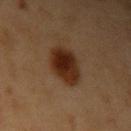{
  "biopsy_status": "not biopsied; imaged during a skin examination",
  "image": {
    "source": "total-body photography crop",
    "field_of_view_mm": 15
  },
  "site": "arm",
  "patient": {
    "sex": "female",
    "age_approx": 40
  },
  "lesion_size": {
    "long_diameter_mm_approx": 4.0
  },
  "automated_metrics": {
    "area_mm2_approx": 12.0,
    "eccentricity": 0.5,
    "shape_asymmetry": 0.1,
    "cielab_L": 23,
    "cielab_a": 17,
    "cielab_b": 25,
    "vs_skin_darker_L": 11.0,
    "border_irregularity_0_10": 1.0,
    "color_variation_0_10": 5.0,
    "peripheral_color_asymmetry": 1.5
  },
  "lighting": "cross-polarized"
}